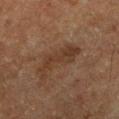A 15 mm close-up tile from a total-body photography series done for melanoma screening. Automated image analysis of the tile measured an eccentricity of roughly 0.9. The analysis additionally found a lesion–skin lightness drop of about 6 and a normalized lesion–skin contrast near 6.5. The analysis additionally found a border-irregularity rating of about 7/10, a color-variation rating of about 2.5/10, and a peripheral color-asymmetry measure near 1. The analysis additionally found a nevus-likeness score of about 0/100. Captured under cross-polarized illumination. From the leg. A male patient approximately 65 years of age.